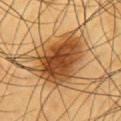Q: Was this lesion biopsied?
A: no biopsy performed (imaged during a skin exam)
Q: What is the anatomic site?
A: the chest
Q: What are the patient's age and sex?
A: male, approximately 60 years of age
Q: What kind of image is this?
A: ~15 mm crop, total-body skin-cancer survey
Q: How was the tile lit?
A: cross-polarized illumination
Q: What is the lesion's diameter?
A: ~7.5 mm (longest diameter)
Q: What did automated image analysis measure?
A: a normalized border contrast of about 11.5